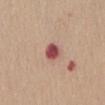Recorded during total-body skin imaging; not selected for excision or biopsy.
A 15 mm crop from a total-body photograph taken for skin-cancer surveillance.
A female subject in their 40s.
The lesion is on the abdomen.
This is a white-light tile.
Measured at roughly 2.5 mm in maximum diameter.